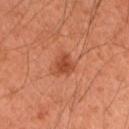No biopsy was performed on this lesion — it was imaged during a full skin examination and was not determined to be concerning.
A 15 mm close-up tile from a total-body photography series done for melanoma screening.
The lesion is on the left upper arm.
A male subject roughly 45 years of age.